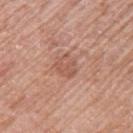| field | value |
|---|---|
| notes | imaged on a skin check; not biopsied |
| lighting | white-light |
| image | ~15 mm crop, total-body skin-cancer survey |
| anatomic site | the left upper arm |
| automated lesion analysis | a nevus-likeness score of about 0/100 and a detector confidence of about 100 out of 100 that the crop contains a lesion |
| patient | female, in their 60s |